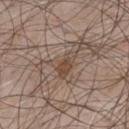Captured during whole-body skin photography for melanoma surveillance; the lesion was not biopsied.
From the chest.
A 15 mm close-up extracted from a 3D total-body photography capture.
A male patient, in their mid- to late 50s.